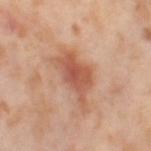The lesion was photographed on a routine skin check and not biopsied; there is no pathology result. A female patient aged approximately 55. A roughly 15 mm field-of-view crop from a total-body skin photograph. Longest diameter approximately 6 mm. Captured under cross-polarized illumination. The total-body-photography lesion software estimated an area of roughly 13 mm², an outline eccentricity of about 0.85 (0 = round, 1 = elongated), and two-axis asymmetry of about 0.4. The software also gave a lesion color around L≈56 a*≈25 b*≈32 in CIELAB, a lesion–skin lightness drop of about 11, and a normalized lesion–skin contrast near 7.5. It also reported a border-irregularity index near 5.5/10, a within-lesion color-variation index near 4/10, and peripheral color asymmetry of about 1.5. On the right thigh.